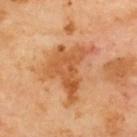Q: Was this lesion biopsied?
A: imaged on a skin check; not biopsied
Q: Illumination type?
A: cross-polarized
Q: Where on the body is the lesion?
A: the upper back
Q: How large is the lesion?
A: about 7 mm
Q: What did automated image analysis measure?
A: a lesion color around L≈55 a*≈26 b*≈41 in CIELAB and a normalized lesion–skin contrast near 7.5; a border-irregularity rating of about 7.5/10, a within-lesion color-variation index near 4/10, and a peripheral color-asymmetry measure near 1.5
Q: What is the imaging modality?
A: 15 mm crop, total-body photography
Q: Patient demographics?
A: male, roughly 70 years of age